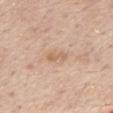notes: total-body-photography surveillance lesion; no biopsy
anatomic site: the mid back
illumination: white-light illumination
acquisition: ~15 mm tile from a whole-body skin photo
patient: male, aged approximately 55